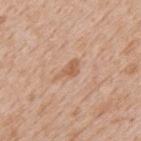Recorded during total-body skin imaging; not selected for excision or biopsy. The lesion is located on the upper back. A male subject, aged around 65. About 3 mm across. Captured under white-light illumination. A roughly 15 mm field-of-view crop from a total-body skin photograph.